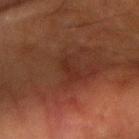- notes — imaged on a skin check; not biopsied
- patient — male, aged approximately 65
- site — the left forearm
- TBP lesion metrics — a border-irregularity rating of about 4/10, a within-lesion color-variation index near 0/10, and a peripheral color-asymmetry measure near 0
- diameter — about 3 mm
- imaging modality — total-body-photography crop, ~15 mm field of view